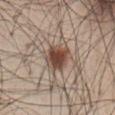- image · total-body-photography crop, ~15 mm field of view
- site · the abdomen
- subject · male, aged around 60